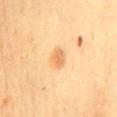Recorded during total-body skin imaging; not selected for excision or biopsy. Approximately 2.5 mm at its widest. Located on the mid back. A female patient in their mid- to late 50s. Imaged with cross-polarized lighting. Automated tile analysis of the lesion measured an area of roughly 3.5 mm², a shape eccentricity near 0.75, and a symmetry-axis asymmetry near 0.3. The software also gave an average lesion color of about L≈60 a*≈19 b*≈38 (CIELAB) and a lesion–skin lightness drop of about 9. The software also gave a border-irregularity index near 2.5/10. The software also gave a classifier nevus-likeness of about 35/100 and lesion-presence confidence of about 100/100. A 15 mm close-up extracted from a 3D total-body photography capture.